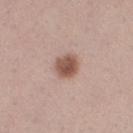  biopsy_status: not biopsied; imaged during a skin examination
  lighting: white-light
  lesion_size:
    long_diameter_mm_approx: 3.0
  image:
    source: total-body photography crop
    field_of_view_mm: 15
  patient:
    sex: female
    age_approx: 40
  site: right thigh